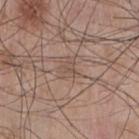{
  "biopsy_status": "not biopsied; imaged during a skin examination",
  "patient": {
    "sex": "male",
    "age_approx": 65
  },
  "lesion_size": {
    "long_diameter_mm_approx": 2.5
  },
  "site": "chest",
  "lighting": "white-light",
  "image": {
    "source": "total-body photography crop",
    "field_of_view_mm": 15
  }
}